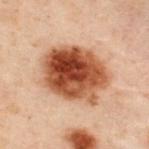Clinical impression:
No biopsy was performed on this lesion — it was imaged during a full skin examination and was not determined to be concerning.
Background:
The lesion's longest dimension is about 7.5 mm. Located on the upper back. A close-up tile cropped from a whole-body skin photograph, about 15 mm across. The subject is a male in their 50s. This is a cross-polarized tile.